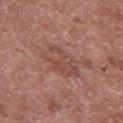Assessment:
Part of a total-body skin-imaging series; this lesion was reviewed on a skin check and was not flagged for biopsy.
Clinical summary:
The patient is a male approximately 65 years of age. Cropped from a total-body skin-imaging series; the visible field is about 15 mm. Located on the right upper arm.